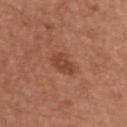This lesion was catalogued during total-body skin photography and was not selected for biopsy. A roughly 15 mm field-of-view crop from a total-body skin photograph. From the upper back. A male subject, aged 63 to 67. The total-body-photography lesion software estimated a lesion area of about 5.5 mm² and a shape-asymmetry score of about 0.25 (0 = symmetric). The analysis additionally found an average lesion color of about L≈44 a*≈25 b*≈31 (CIELAB). The analysis additionally found border irregularity of about 2 on a 0–10 scale, internal color variation of about 2.5 on a 0–10 scale, and radial color variation of about 1. Imaged with white-light lighting. The recorded lesion diameter is about 3 mm.